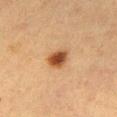Part of a total-body skin-imaging series; this lesion was reviewed on a skin check and was not flagged for biopsy. A female patient, aged around 55. From the right thigh. About 3 mm across. A roughly 15 mm field-of-view crop from a total-body skin photograph. This is a cross-polarized tile. Automated tile analysis of the lesion measured lesion-presence confidence of about 100/100.